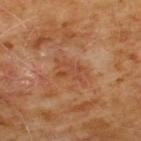follow-up=imaged on a skin check; not biopsied
location=the chest
TBP lesion metrics=a footprint of about 5.5 mm² and two-axis asymmetry of about 0.4; a lesion color around L≈45 a*≈24 b*≈34 in CIELAB and a normalized lesion–skin contrast near 5.5; a border-irregularity rating of about 5.5/10, a within-lesion color-variation index near 1.5/10, and radial color variation of about 0.5; a classifier nevus-likeness of about 0/100 and lesion-presence confidence of about 100/100
diameter=~4 mm (longest diameter)
image=15 mm crop, total-body photography
subject=male, aged approximately 60
tile lighting=cross-polarized illumination